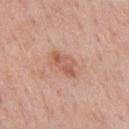Case summary:
- notes — catalogued during a skin exam; not biopsied
- image source — ~15 mm tile from a whole-body skin photo
- lighting — white-light
- lesion diameter — ≈3.5 mm
- image-analysis metrics — an area of roughly 5 mm²; a within-lesion color-variation index near 4/10 and a peripheral color-asymmetry measure near 1; a classifier nevus-likeness of about 5/100 and lesion-presence confidence of about 100/100
- subject — male, roughly 55 years of age
- location — the chest